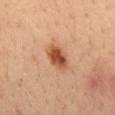The lesion was tiled from a total-body skin photograph and was not biopsied.
The recorded lesion diameter is about 3.5 mm.
The lesion-visualizer software estimated an outline eccentricity of about 0.8 (0 = round, 1 = elongated). And it measured an average lesion color of about L≈47 a*≈24 b*≈33 (CIELAB), about 14 CIELAB-L* units darker than the surrounding skin, and a normalized lesion–skin contrast near 10. The software also gave a border-irregularity index near 1.5/10 and peripheral color asymmetry of about 1. It also reported a lesion-detection confidence of about 100/100.
A 15 mm close-up extracted from a 3D total-body photography capture.
The tile uses cross-polarized illumination.
A male subject, about 35 years old.
On the mid back.